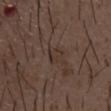Captured during whole-body skin photography for melanoma surveillance; the lesion was not biopsied. A male patient, aged 48–52. The tile uses white-light illumination. The lesion-visualizer software estimated border irregularity of about 3 on a 0–10 scale, internal color variation of about 2.5 on a 0–10 scale, and radial color variation of about 1. Longest diameter approximately 2.5 mm. On the front of the torso. A lesion tile, about 15 mm wide, cut from a 3D total-body photograph.